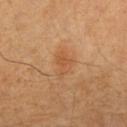This lesion was catalogued during total-body skin photography and was not selected for biopsy.
The tile uses cross-polarized illumination.
A female patient aged 48–52.
On the arm.
A 15 mm close-up tile from a total-body photography series done for melanoma screening.
About 3 mm across.